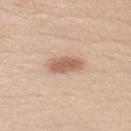  biopsy_status: not biopsied; imaged during a skin examination
  patient:
    sex: female
    age_approx: 35
  site: back
  lighting: white-light
  lesion_size:
    long_diameter_mm_approx: 4.0
  image:
    source: total-body photography crop
    field_of_view_mm: 15